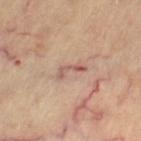<case>
  <biopsy_status>not biopsied; imaged during a skin examination</biopsy_status>
  <automated_metrics>
    <cielab_L>57</cielab_L>
    <cielab_a>22</cielab_a>
    <cielab_b>24</cielab_b>
    <vs_skin_darker_L>10.0</vs_skin_darker_L>
    <vs_skin_contrast_norm>7.5</vs_skin_contrast_norm>
    <nevus_likeness_0_100>0</nevus_likeness_0_100>
  </automated_metrics>
  <site>right leg</site>
  <image>
    <source>total-body photography crop</source>
    <field_of_view_mm>15</field_of_view_mm>
  </image>
  <lighting>cross-polarized</lighting>
  <lesion_size>
    <long_diameter_mm_approx>3.5</long_diameter_mm_approx>
  </lesion_size>
  <patient>
    <sex>female</sex>
    <age_approx>60</age_approx>
  </patient>
</case>